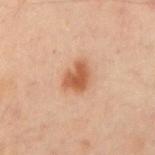Clinical impression: Captured during whole-body skin photography for melanoma surveillance; the lesion was not biopsied. Background: A male subject, aged approximately 50. From the right upper arm. Imaged with cross-polarized lighting. A lesion tile, about 15 mm wide, cut from a 3D total-body photograph. The total-body-photography lesion software estimated border irregularity of about 2.5 on a 0–10 scale and a within-lesion color-variation index near 3/10. The software also gave an automated nevus-likeness rating near 100 out of 100. The lesion's longest dimension is about 3.5 mm.